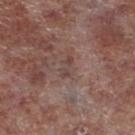Captured during whole-body skin photography for melanoma surveillance; the lesion was not biopsied.
The subject is a male roughly 55 years of age.
From the leg.
A lesion tile, about 15 mm wide, cut from a 3D total-body photograph.
The tile uses white-light illumination.
About 2.5 mm across.
An algorithmic analysis of the crop reported a footprint of about 2.5 mm², a shape eccentricity near 0.9, and a symmetry-axis asymmetry near 0.45. The analysis additionally found a border-irregularity index near 5.5/10 and a within-lesion color-variation index near 0/10. It also reported a nevus-likeness score of about 0/100 and lesion-presence confidence of about 90/100.